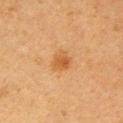Q: Was this lesion biopsied?
A: catalogued during a skin exam; not biopsied
Q: Where on the body is the lesion?
A: the right upper arm
Q: What is the imaging modality?
A: 15 mm crop, total-body photography
Q: Patient demographics?
A: female, aged 53 to 57